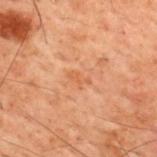Q: Is there a histopathology result?
A: imaged on a skin check; not biopsied
Q: Where on the body is the lesion?
A: the back
Q: Who is the patient?
A: male, aged 58 to 62
Q: What lighting was used for the tile?
A: cross-polarized
Q: How was this image acquired?
A: ~15 mm crop, total-body skin-cancer survey